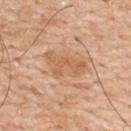The lesion was tiled from a total-body skin photograph and was not biopsied. Approximately 5.5 mm at its widest. On the upper back. The subject is a male aged around 60. The total-body-photography lesion software estimated a border-irregularity rating of about 4.5/10, internal color variation of about 2.5 on a 0–10 scale, and peripheral color asymmetry of about 1. Captured under white-light illumination. A lesion tile, about 15 mm wide, cut from a 3D total-body photograph.